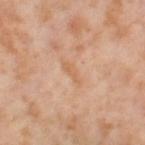{"biopsy_status": "not biopsied; imaged during a skin examination", "patient": {"sex": "female", "age_approx": 55}, "image": {"source": "total-body photography crop", "field_of_view_mm": 15}, "lesion_size": {"long_diameter_mm_approx": 3.5}, "site": "right thigh", "lighting": "cross-polarized"}